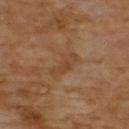This image is a 15 mm lesion crop taken from a total-body photograph. A male subject about 70 years old. Longest diameter approximately 4 mm. Captured under cross-polarized illumination. Located on the back. An algorithmic analysis of the crop reported a lesion color around L≈42 a*≈19 b*≈33 in CIELAB and a lesion-to-skin contrast of about 5.5 (normalized; higher = more distinct). And it measured internal color variation of about 2 on a 0–10 scale and peripheral color asymmetry of about 0.5.